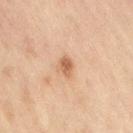automated lesion analysis — a within-lesion color-variation index near 2/10 and a peripheral color-asymmetry measure near 0.5; lesion diameter — about 2.5 mm; subject — female, aged approximately 60; location — the lower back; acquisition — ~15 mm tile from a whole-body skin photo; tile lighting — cross-polarized illumination.